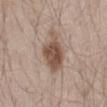Case summary:
- biopsy status · catalogued during a skin exam; not biopsied
- body site · the mid back
- automated metrics · an area of roughly 13 mm², an outline eccentricity of about 0.8 (0 = round, 1 = elongated), and a shape-asymmetry score of about 0.25 (0 = symmetric); border irregularity of about 3 on a 0–10 scale, a color-variation rating of about 3.5/10, and peripheral color asymmetry of about 1
- subject · male, aged 38 to 42
- lesion size · ~5.5 mm (longest diameter)
- image · 15 mm crop, total-body photography
- illumination · white-light illumination